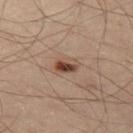This is a cross-polarized tile. A male subject aged 48 to 52. Approximately 3 mm at its widest. Located on the left thigh. The total-body-photography lesion software estimated border irregularity of about 2 on a 0–10 scale, internal color variation of about 8.5 on a 0–10 scale, and a peripheral color-asymmetry measure near 3. The software also gave an automated nevus-likeness rating near 95 out of 100 and a lesion-detection confidence of about 100/100. A lesion tile, about 15 mm wide, cut from a 3D total-body photograph.